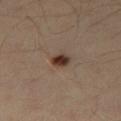Notes:
• patient · male, in their 40s
• lesion size · ≈2.5 mm
• acquisition · ~15 mm tile from a whole-body skin photo
• site · the right thigh
• lighting · cross-polarized